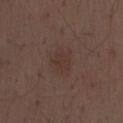Recorded during total-body skin imaging; not selected for excision or biopsy. A 15 mm close-up extracted from a 3D total-body photography capture. A male subject approximately 50 years of age.